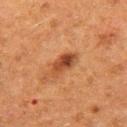biopsy_status: not biopsied; imaged during a skin examination
site: left thigh
image:
  source: total-body photography crop
  field_of_view_mm: 15
patient:
  sex: female
  age_approx: 50